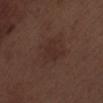Recorded during total-body skin imaging; not selected for excision or biopsy.
The lesion is located on the leg.
The subject is a male aged 68 to 72.
The recorded lesion diameter is about 5.5 mm.
This is a white-light tile.
A 15 mm close-up extracted from a 3D total-body photography capture.
An algorithmic analysis of the crop reported a footprint of about 16 mm², a shape eccentricity near 0.7, and a symmetry-axis asymmetry near 0.2. And it measured a border-irregularity rating of about 2/10, internal color variation of about 2 on a 0–10 scale, and a peripheral color-asymmetry measure near 0.5. It also reported a classifier nevus-likeness of about 5/100 and lesion-presence confidence of about 100/100.